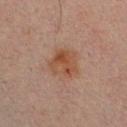Automated tile analysis of the lesion measured a lesion area of about 11 mm², an outline eccentricity of about 0.3 (0 = round, 1 = elongated), and a symmetry-axis asymmetry near 0.2. The software also gave a mean CIELAB color near L≈40 a*≈18 b*≈26, roughly 6 lightness units darker than nearby skin, and a normalized border contrast of about 7.5. The analysis additionally found a border-irregularity index near 2/10, a color-variation rating of about 5/10, and peripheral color asymmetry of about 1.5.
Cropped from a whole-body photographic skin survey; the tile spans about 15 mm.
Located on the chest.
The lesion's longest dimension is about 4 mm.
The subject is a male about 30 years old.
Imaged with cross-polarized lighting.